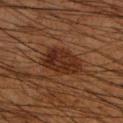Imaged during a routine full-body skin examination; the lesion was not biopsied and no histopathology is available. An algorithmic analysis of the crop reported an average lesion color of about L≈22 a*≈18 b*≈24 (CIELAB) and about 8 CIELAB-L* units darker than the surrounding skin. And it measured a lesion-detection confidence of about 100/100. The lesion is located on the right upper arm. A close-up tile cropped from a whole-body skin photograph, about 15 mm across. The lesion's longest dimension is about 5 mm. The tile uses cross-polarized illumination. A male patient, about 65 years old.